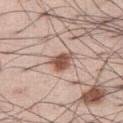Q: Was a biopsy performed?
A: no biopsy performed (imaged during a skin exam)
Q: Who is the patient?
A: male, in their mid- to late 50s
Q: How was this image acquired?
A: 15 mm crop, total-body photography
Q: What lighting was used for the tile?
A: white-light illumination
Q: Lesion location?
A: the leg